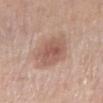{"biopsy_status": "not biopsied; imaged during a skin examination", "image": {"source": "total-body photography crop", "field_of_view_mm": 15}, "lesion_size": {"long_diameter_mm_approx": 4.5}, "site": "right lower leg", "lighting": "white-light", "patient": {"sex": "female", "age_approx": 65}}